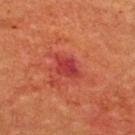follow-up: imaged on a skin check; not biopsied
image source: ~15 mm tile from a whole-body skin photo
patient: male, aged 63–67
location: the chest
tile lighting: cross-polarized illumination
size: ≈3.5 mm
TBP lesion metrics: an area of roughly 6 mm² and a symmetry-axis asymmetry near 0.25; about 9 CIELAB-L* units darker than the surrounding skin and a normalized border contrast of about 7.5; border irregularity of about 3 on a 0–10 scale and a within-lesion color-variation index near 5/10; an automated nevus-likeness rating near 25 out of 100